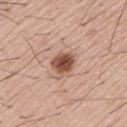The lesion was tiled from a total-body skin photograph and was not biopsied. This image is a 15 mm lesion crop taken from a total-body photograph. Measured at roughly 3 mm in maximum diameter. Automated image analysis of the tile measured an area of roughly 6.5 mm², a shape eccentricity near 0.5, and a shape-asymmetry score of about 0.2 (0 = symmetric). The software also gave an average lesion color of about L≈51 a*≈22 b*≈29 (CIELAB), about 16 CIELAB-L* units darker than the surrounding skin, and a lesion-to-skin contrast of about 11 (normalized; higher = more distinct). It also reported an automated nevus-likeness rating near 100 out of 100 and lesion-presence confidence of about 100/100. A male patient roughly 55 years of age. Imaged with white-light lighting.